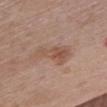{"biopsy_status": "not biopsied; imaged during a skin examination", "image": {"source": "total-body photography crop", "field_of_view_mm": 15}, "patient": {"sex": "female", "age_approx": 80}, "site": "chest", "lesion_size": {"long_diameter_mm_approx": 5.0}}